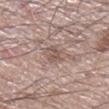Clinical impression:
This lesion was catalogued during total-body skin photography and was not selected for biopsy.
Image and clinical context:
Imaged with white-light lighting. A male patient roughly 60 years of age. A close-up tile cropped from a whole-body skin photograph, about 15 mm across. Longest diameter approximately 3 mm. The lesion is located on the leg.